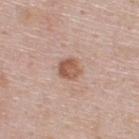No biopsy was performed on this lesion — it was imaged during a full skin examination and was not determined to be concerning.
A male patient about 45 years old.
About 2.5 mm across.
This is a white-light tile.
The lesion is located on the upper back.
A 15 mm close-up extracted from a 3D total-body photography capture.